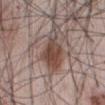workup: total-body-photography surveillance lesion; no biopsy | patient: male, about 45 years old | body site: the abdomen | acquisition: 15 mm crop, total-body photography | illumination: white-light | automated metrics: a lesion area of about 12 mm², an eccentricity of roughly 0.85, and a symmetry-axis asymmetry near 0.3; roughly 12 lightness units darker than nearby skin and a lesion-to-skin contrast of about 9 (normalized; higher = more distinct); border irregularity of about 4 on a 0–10 scale and a peripheral color-asymmetry measure near 1.5; a classifier nevus-likeness of about 95/100 and lesion-presence confidence of about 100/100.